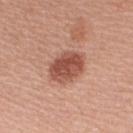Q: Was this lesion biopsied?
A: total-body-photography surveillance lesion; no biopsy
Q: Lesion location?
A: the right upper arm
Q: What kind of image is this?
A: ~15 mm tile from a whole-body skin photo
Q: Who is the patient?
A: female, aged around 60